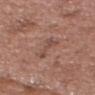Assessment:
Part of a total-body skin-imaging series; this lesion was reviewed on a skin check and was not flagged for biopsy.
Context:
A close-up tile cropped from a whole-body skin photograph, about 15 mm across. On the head or neck. The patient is a male approximately 55 years of age.